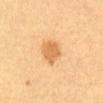workup: catalogued during a skin exam; not biopsied | illumination: cross-polarized illumination | diameter: ~3.5 mm (longest diameter) | acquisition: ~15 mm tile from a whole-body skin photo | location: the abdomen | patient: female, aged 18 to 22.